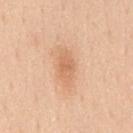workup: total-body-photography surveillance lesion; no biopsy
imaging modality: 15 mm crop, total-body photography
subject: male, aged 48–52
size: about 3.5 mm
illumination: white-light illumination
location: the chest